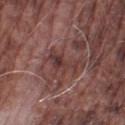Q: Was this lesion biopsied?
A: catalogued during a skin exam; not biopsied
Q: How was this image acquired?
A: ~15 mm crop, total-body skin-cancer survey
Q: Lesion location?
A: the right lower leg
Q: What lighting was used for the tile?
A: white-light illumination
Q: Who is the patient?
A: male, aged 73 to 77